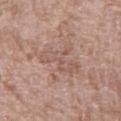  biopsy_status: not biopsied; imaged during a skin examination
  patient:
    sex: female
    age_approx: 70
  site: arm
  image:
    source: total-body photography crop
    field_of_view_mm: 15